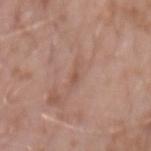Imaged during a routine full-body skin examination; the lesion was not biopsied and no histopathology is available. An algorithmic analysis of the crop reported roughly 7 lightness units darker than nearby skin and a lesion-to-skin contrast of about 5 (normalized; higher = more distinct). The software also gave peripheral color asymmetry of about 0. And it measured an automated nevus-likeness rating near 0 out of 100. The lesion is located on the right forearm. Captured under white-light illumination. A close-up tile cropped from a whole-body skin photograph, about 15 mm across. A male subject, about 60 years old.